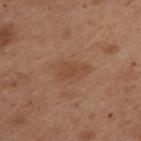- biopsy status · imaged on a skin check; not biopsied
- imaging modality · ~15 mm crop, total-body skin-cancer survey
- anatomic site · the upper back
- automated metrics · a mean CIELAB color near L≈38 a*≈17 b*≈26 and a lesion-to-skin contrast of about 5 (normalized; higher = more distinct); border irregularity of about 3 on a 0–10 scale, a within-lesion color-variation index near 2/10, and a peripheral color-asymmetry measure near 0.5; a classifier nevus-likeness of about 0/100
- diameter · about 4 mm
- lighting · cross-polarized
- subject · female, in their mid- to late 50s